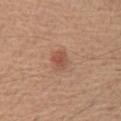{"lighting": "white-light", "patient": {"sex": "male", "age_approx": 65}, "image": {"source": "total-body photography crop", "field_of_view_mm": 15}, "lesion_size": {"long_diameter_mm_approx": 3.0}, "automated_metrics": {"cielab_L": 52, "cielab_a": 23, "cielab_b": 30, "vs_skin_darker_L": 9.0, "vs_skin_contrast_norm": 6.0}, "site": "chest"}